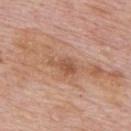workup = imaged on a skin check; not biopsied
patient = male, approximately 75 years of age
anatomic site = the back
illumination = white-light illumination
image = ~15 mm crop, total-body skin-cancer survey
lesion size = about 3.5 mm